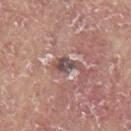<lesion>
<biopsy_status>not biopsied; imaged during a skin examination</biopsy_status>
<patient>
  <sex>male</sex>
  <age_approx>65</age_approx>
</patient>
<site>left upper arm</site>
<lesion_size>
  <long_diameter_mm_approx>3.0</long_diameter_mm_approx>
</lesion_size>
<image>
  <source>total-body photography crop</source>
  <field_of_view_mm>15</field_of_view_mm>
</image>
<lighting>white-light</lighting>
<automated_metrics>
  <border_irregularity_0_10>3.5</border_irregularity_0_10>
  <nevus_likeness_0_100>0</nevus_likeness_0_100>
  <lesion_detection_confidence_0_100>90</lesion_detection_confidence_0_100>
</automated_metrics>
</lesion>